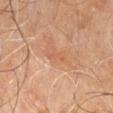biopsy_status: not biopsied; imaged during a skin examination
site: abdomen
image:
  source: total-body photography crop
  field_of_view_mm: 15
patient:
  sex: male
  age_approx: 60